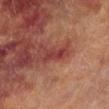Impression: Captured during whole-body skin photography for melanoma surveillance; the lesion was not biopsied. Image and clinical context: A male subject aged 68 to 72. Approximately 3 mm at its widest. A close-up tile cropped from a whole-body skin photograph, about 15 mm across. On the right lower leg. Captured under cross-polarized illumination.